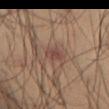Assessment: This lesion was catalogued during total-body skin photography and was not selected for biopsy. Image and clinical context: Located on the leg. The subject is a male aged 38 to 42. This is a cross-polarized tile. A 15 mm crop from a total-body photograph taken for skin-cancer surveillance. Automated tile analysis of the lesion measured about 7 CIELAB-L* units darker than the surrounding skin and a normalized lesion–skin contrast near 6. The software also gave border irregularity of about 3 on a 0–10 scale. About 2.5 mm across.